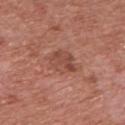{"biopsy_status": "not biopsied; imaged during a skin examination", "image": {"source": "total-body photography crop", "field_of_view_mm": 15}, "lesion_size": {"long_diameter_mm_approx": 4.0}, "site": "front of the torso", "patient": {"sex": "male", "age_approx": 75}, "lighting": "white-light"}